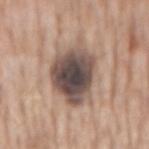<lesion>
<image>
  <source>total-body photography crop</source>
  <field_of_view_mm>15</field_of_view_mm>
</image>
<patient>
  <sex>male</sex>
  <age_approx>60</age_approx>
</patient>
<site>front of the torso</site>
</lesion>